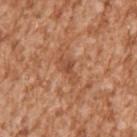| key | value |
|---|---|
| biopsy status | total-body-photography surveillance lesion; no biopsy |
| anatomic site | the right upper arm |
| imaging modality | ~15 mm crop, total-body skin-cancer survey |
| patient | male, aged 43 to 47 |
| lighting | white-light illumination |